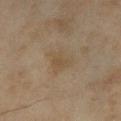This lesion was catalogued during total-body skin photography and was not selected for biopsy.
The lesion is on the left lower leg.
A 15 mm close-up tile from a total-body photography series done for melanoma screening.
The subject is a female in their 60s.